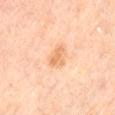The lesion was photographed on a routine skin check and not biopsied; there is no pathology result. Automated tile analysis of the lesion measured a lesion color around L≈71 a*≈22 b*≈40 in CIELAB, about 9 CIELAB-L* units darker than the surrounding skin, and a normalized border contrast of about 6.5. And it measured border irregularity of about 4 on a 0–10 scale, internal color variation of about 2 on a 0–10 scale, and radial color variation of about 0.5. It also reported an automated nevus-likeness rating near 20 out of 100 and a lesion-detection confidence of about 100/100. About 2.5 mm across. The lesion is on the left thigh. This image is a 15 mm lesion crop taken from a total-body photograph. A male subject, roughly 70 years of age.